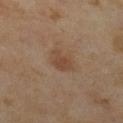<record>
  <biopsy_status>not biopsied; imaged during a skin examination</biopsy_status>
  <patient>
    <sex>female</sex>
    <age_approx>60</age_approx>
  </patient>
  <site>right lower leg</site>
  <image>
    <source>total-body photography crop</source>
    <field_of_view_mm>15</field_of_view_mm>
  </image>
</record>